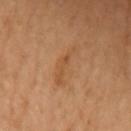  biopsy_status: not biopsied; imaged during a skin examination
  site: chest
  patient:
    sex: female
    age_approx: 60
  image:
    source: total-body photography crop
    field_of_view_mm: 15
  lighting: cross-polarized
  lesion_size:
    long_diameter_mm_approx: 5.0
  automated_metrics:
    cielab_L: 44
    cielab_a: 19
    cielab_b: 33
    vs_skin_darker_L: 5.0
    vs_skin_contrast_norm: 4.5